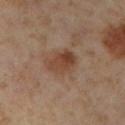<case>
  <biopsy_status>not biopsied; imaged during a skin examination</biopsy_status>
  <patient>
    <sex>female</sex>
    <age_approx>55</age_approx>
  </patient>
  <image>
    <source>total-body photography crop</source>
    <field_of_view_mm>15</field_of_view_mm>
  </image>
  <site>right lower leg</site>
</case>